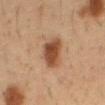Part of a total-body skin-imaging series; this lesion was reviewed on a skin check and was not flagged for biopsy. The recorded lesion diameter is about 4.5 mm. A lesion tile, about 15 mm wide, cut from a 3D total-body photograph. The subject is a male aged approximately 50. Located on the abdomen.